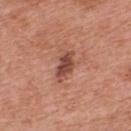Impression: Captured during whole-body skin photography for melanoma surveillance; the lesion was not biopsied. Background: From the upper back. Cropped from a whole-body photographic skin survey; the tile spans about 15 mm. A male subject, aged around 70.